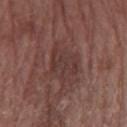Part of a total-body skin-imaging series; this lesion was reviewed on a skin check and was not flagged for biopsy.
Imaged with white-light lighting.
From the right forearm.
The lesion's longest dimension is about 4 mm.
The subject is a female approximately 70 years of age.
A close-up tile cropped from a whole-body skin photograph, about 15 mm across.